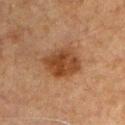Assessment:
Part of a total-body skin-imaging series; this lesion was reviewed on a skin check and was not flagged for biopsy.
Image and clinical context:
About 4.5 mm across. On the chest. Cropped from a total-body skin-imaging series; the visible field is about 15 mm. A male subject, approximately 50 years of age. The lesion-visualizer software estimated a shape eccentricity near 0.55 and two-axis asymmetry of about 0.15. The analysis additionally found a mean CIELAB color near L≈34 a*≈19 b*≈29, a lesion–skin lightness drop of about 10, and a normalized border contrast of about 9.5.